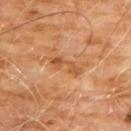Q: Was a biopsy performed?
A: catalogued during a skin exam; not biopsied
Q: What are the patient's age and sex?
A: male, aged around 60
Q: Where on the body is the lesion?
A: the chest
Q: What kind of image is this?
A: total-body-photography crop, ~15 mm field of view